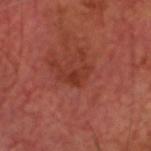  biopsy_status: not biopsied; imaged during a skin examination
  automated_metrics:
    cielab_L: 33
    cielab_a: 28
    cielab_b: 29
    vs_skin_darker_L: 6.0
    vs_skin_contrast_norm: 5.5
  lighting: cross-polarized
  site: head or neck
  image:
    source: total-body photography crop
    field_of_view_mm: 15
  lesion_size:
    long_diameter_mm_approx: 3.0
  patient:
    sex: male
    age_approx: 65